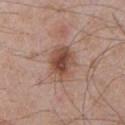workup=catalogued during a skin exam; not biopsied
anatomic site=the front of the torso
TBP lesion metrics=a lesion area of about 11 mm², a shape eccentricity near 0.7, and two-axis asymmetry of about 0.2; a border-irregularity index near 2.5/10 and a peripheral color-asymmetry measure near 1.5; a nevus-likeness score of about 70/100 and a detector confidence of about 100 out of 100 that the crop contains a lesion
diameter=about 4.5 mm
patient=male, aged 63–67
acquisition=15 mm crop, total-body photography